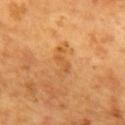| field | value |
|---|---|
| follow-up | no biopsy performed (imaged during a skin exam) |
| patient | male, aged 63 to 67 |
| image-analysis metrics | a lesion area of about 4 mm², an eccentricity of roughly 0.9, and two-axis asymmetry of about 0.5; a border-irregularity rating of about 6.5/10, internal color variation of about 0 on a 0–10 scale, and radial color variation of about 0 |
| body site | the upper back |
| acquisition | 15 mm crop, total-body photography |